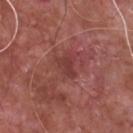Clinical summary: A male subject, in their mid-60s. The lesion is on the chest. A 15 mm crop from a total-body photograph taken for skin-cancer surveillance.